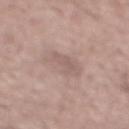Background:
A close-up tile cropped from a whole-body skin photograph, about 15 mm across. Approximately 4 mm at its widest. The lesion-visualizer software estimated a mean CIELAB color near L≈58 a*≈16 b*≈21 and roughly 7 lightness units darker than nearby skin. The analysis additionally found border irregularity of about 3.5 on a 0–10 scale and a color-variation rating of about 1.5/10. A male patient, aged approximately 55. The lesion is on the mid back.